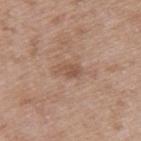This lesion was catalogued during total-body skin photography and was not selected for biopsy. Captured under white-light illumination. This image is a 15 mm lesion crop taken from a total-body photograph. A male patient about 50 years old. From the left upper arm.